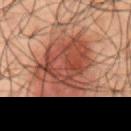biopsy status = total-body-photography surveillance lesion; no biopsy
image = total-body-photography crop, ~15 mm field of view
patient = male, approximately 50 years of age
lesion diameter = ≈9 mm
automated metrics = a lesion color around L≈37 a*≈20 b*≈24 in CIELAB and a lesion–skin lightness drop of about 10; an automated nevus-likeness rating near 80 out of 100
anatomic site = the abdomen